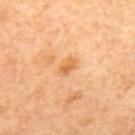No biopsy was performed on this lesion — it was imaged during a full skin examination and was not determined to be concerning. The patient is a female aged around 65. The lesion's longest dimension is about 2.5 mm. The total-body-photography lesion software estimated an area of roughly 3.5 mm², an eccentricity of roughly 0.8, and a shape-asymmetry score of about 0.3 (0 = symmetric). Located on the back. A 15 mm close-up tile from a total-body photography series done for melanoma screening. This is a cross-polarized tile.